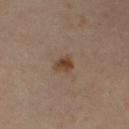biopsy_status: not biopsied; imaged during a skin examination
patient:
  sex: female
  age_approx: 40
lighting: cross-polarized
lesion_size:
  long_diameter_mm_approx: 3.0
site: right thigh
automated_metrics:
  area_mm2_approx: 4.0
  eccentricity: 0.7
  shape_asymmetry: 0.3
  nevus_likeness_0_100: 85
  lesion_detection_confidence_0_100: 100
image:
  source: total-body photography crop
  field_of_view_mm: 15